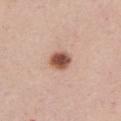The lesion was photographed on a routine skin check and not biopsied; there is no pathology result. The recorded lesion diameter is about 3 mm. A female subject, aged around 65. On the chest. Imaged with white-light lighting. A 15 mm crop from a total-body photograph taken for skin-cancer surveillance.